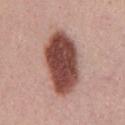A male subject in their mid- to late 30s.
A region of skin cropped from a whole-body photographic capture, roughly 15 mm wide.
The lesion's longest dimension is about 8 mm.
The lesion-visualizer software estimated a lesion area of about 28 mm² and two-axis asymmetry of about 0.15. It also reported roughly 21 lightness units darker than nearby skin and a normalized border contrast of about 13.5. The software also gave a classifier nevus-likeness of about 100/100 and a lesion-detection confidence of about 100/100.
Located on the chest.